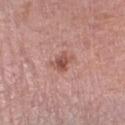Recorded during total-body skin imaging; not selected for excision or biopsy. A roughly 15 mm field-of-view crop from a total-body skin photograph. A female subject, approximately 70 years of age. From the right lower leg. The lesion's longest dimension is about 3 mm.